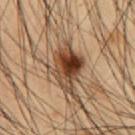Findings:
* image: total-body-photography crop, ~15 mm field of view
* body site: the abdomen
* subject: male, aged 53–57